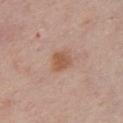Assessment:
Part of a total-body skin-imaging series; this lesion was reviewed on a skin check and was not flagged for biopsy.
Clinical summary:
The subject is a male about 60 years old. Captured under white-light illumination. The lesion's longest dimension is about 2.5 mm. A close-up tile cropped from a whole-body skin photograph, about 15 mm across. The lesion is on the chest.